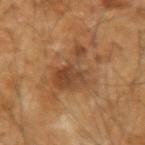Clinical impression: Imaged during a routine full-body skin examination; the lesion was not biopsied and no histopathology is available. Clinical summary: A male patient in their mid- to late 50s. The total-body-photography lesion software estimated a lesion color around L≈41 a*≈19 b*≈32 in CIELAB. And it measured a nevus-likeness score of about 0/100 and lesion-presence confidence of about 100/100. The lesion is on the left forearm. Longest diameter approximately 5.5 mm. This is a cross-polarized tile. A close-up tile cropped from a whole-body skin photograph, about 15 mm across.